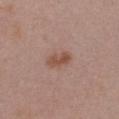Case summary:
– biopsy status · catalogued during a skin exam; not biopsied
– location · the chest
– imaging modality · ~15 mm crop, total-body skin-cancer survey
– subject · female, aged 28–32